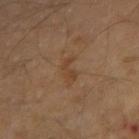An algorithmic analysis of the crop reported a lesion area of about 3 mm², an outline eccentricity of about 0.9 (0 = round, 1 = elongated), and a symmetry-axis asymmetry near 0.45. And it measured a border-irregularity index near 5.5/10. The analysis additionally found a classifier nevus-likeness of about 0/100. Imaged with cross-polarized lighting. The lesion is located on the right forearm. Approximately 2.5 mm at its widest. A 15 mm crop from a total-body photograph taken for skin-cancer surveillance. A male patient, about 70 years old.